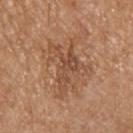Captured during whole-body skin photography for melanoma surveillance; the lesion was not biopsied. A roughly 15 mm field-of-view crop from a total-body skin photograph. The tile uses white-light illumination. A male patient aged 68–72. An algorithmic analysis of the crop reported an area of roughly 12 mm², an outline eccentricity of about 0.8 (0 = round, 1 = elongated), and two-axis asymmetry of about 0.65. And it measured an average lesion color of about L≈49 a*≈21 b*≈32 (CIELAB), about 9 CIELAB-L* units darker than the surrounding skin, and a normalized border contrast of about 6.5. It also reported internal color variation of about 3.5 on a 0–10 scale and radial color variation of about 1.5. The analysis additionally found lesion-presence confidence of about 100/100. From the chest.